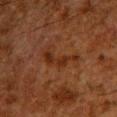{
  "biopsy_status": "not biopsied; imaged during a skin examination",
  "lesion_size": {
    "long_diameter_mm_approx": 5.5
  },
  "patient": {
    "sex": "male",
    "age_approx": 60
  },
  "site": "chest",
  "lighting": "cross-polarized",
  "image": {
    "source": "total-body photography crop",
    "field_of_view_mm": 15
  }
}